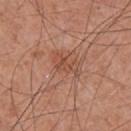Assessment: This lesion was catalogued during total-body skin photography and was not selected for biopsy. Clinical summary: A male patient aged approximately 55. A 15 mm close-up extracted from a 3D total-body photography capture. This is a white-light tile. The lesion is on the chest. Approximately 3.5 mm at its widest.